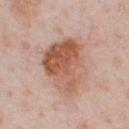Assessment:
This lesion was catalogued during total-body skin photography and was not selected for biopsy.
Acquisition and patient details:
A 15 mm close-up extracted from a 3D total-body photography capture. The lesion is located on the chest. The tile uses white-light illumination. A male subject about 60 years old. The total-body-photography lesion software estimated an average lesion color of about L≈56 a*≈23 b*≈30 (CIELAB), a lesion–skin lightness drop of about 12, and a lesion-to-skin contrast of about 9 (normalized; higher = more distinct). The software also gave a lesion-detection confidence of about 100/100. Approximately 7 mm at its widest.